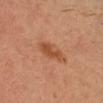{"biopsy_status": "not biopsied; imaged during a skin examination", "lesion_size": {"long_diameter_mm_approx": 3.0}, "lighting": "cross-polarized", "patient": {"sex": "male", "age_approx": 35}, "site": "head or neck", "image": {"source": "total-body photography crop", "field_of_view_mm": 15}}